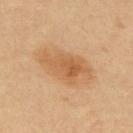Impression: Part of a total-body skin-imaging series; this lesion was reviewed on a skin check and was not flagged for biopsy. Context: On the back. This image is a 15 mm lesion crop taken from a total-body photograph. Captured under cross-polarized illumination. The recorded lesion diameter is about 5.5 mm. A male patient, aged 53–57.